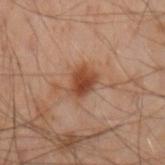Impression: Captured during whole-body skin photography for melanoma surveillance; the lesion was not biopsied. Clinical summary: The total-body-photography lesion software estimated an area of roughly 7 mm², an outline eccentricity of about 0.6 (0 = round, 1 = elongated), and a symmetry-axis asymmetry near 0.25. And it measured an average lesion color of about L≈36 a*≈19 b*≈27 (CIELAB), about 10 CIELAB-L* units darker than the surrounding skin, and a lesion-to-skin contrast of about 9 (normalized; higher = more distinct). A 15 mm close-up tile from a total-body photography series done for melanoma screening. Located on the left arm. Longest diameter approximately 3.5 mm. The subject is a male aged around 50.